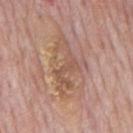Imaged during a routine full-body skin examination; the lesion was not biopsied and no histopathology is available. From the mid back. The subject is a male approximately 75 years of age. A 15 mm close-up extracted from a 3D total-body photography capture.